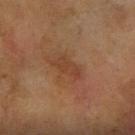Notes:
- follow-up — imaged on a skin check; not biopsied
- diameter — about 3.5 mm
- patient — female, aged 58 to 62
- image — total-body-photography crop, ~15 mm field of view
- illumination — cross-polarized
- anatomic site — the right forearm